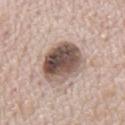The lesion was tiled from a total-body skin photograph and was not biopsied. Imaged with white-light lighting. The patient is a male aged approximately 70. From the mid back. Longest diameter approximately 6 mm. Cropped from a total-body skin-imaging series; the visible field is about 15 mm. Automated tile analysis of the lesion measured a lesion area of about 19 mm² and a shape-asymmetry score of about 0.1 (0 = symmetric). It also reported a lesion color around L≈51 a*≈15 b*≈22 in CIELAB and about 19 CIELAB-L* units darker than the surrounding skin.